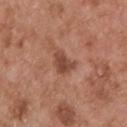Recorded during total-body skin imaging; not selected for excision or biopsy. Imaged with white-light lighting. A male patient roughly 55 years of age. A roughly 15 mm field-of-view crop from a total-body skin photograph. The lesion is on the upper back. Automated image analysis of the tile measured a lesion area of about 5 mm², an eccentricity of roughly 0.55, and two-axis asymmetry of about 0.4.